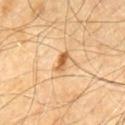follow-up — no biopsy performed (imaged during a skin exam)
imaging modality — 15 mm crop, total-body photography
site — the chest
size — about 3 mm
illumination — cross-polarized
automated metrics — a lesion color around L≈60 a*≈22 b*≈42 in CIELAB, roughly 13 lightness units darker than nearby skin, and a lesion-to-skin contrast of about 9 (normalized; higher = more distinct); a border-irregularity index near 2.5/10, a within-lesion color-variation index near 4/10, and a peripheral color-asymmetry measure near 1; a nevus-likeness score of about 70/100 and a lesion-detection confidence of about 100/100
subject — male, aged around 60